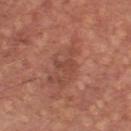Case summary:
• image source · ~15 mm crop, total-body skin-cancer survey
• location · the chest
• automated lesion analysis · a nevus-likeness score of about 0/100 and a lesion-detection confidence of about 100/100
• illumination · white-light
• diameter · ~2.5 mm (longest diameter)
• patient · male, roughly 65 years of age